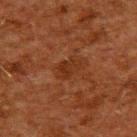Q: Was this lesion biopsied?
A: total-body-photography surveillance lesion; no biopsy
Q: What kind of image is this?
A: 15 mm crop, total-body photography
Q: Illumination type?
A: cross-polarized
Q: Where on the body is the lesion?
A: the upper back
Q: Patient demographics?
A: male, in their 60s